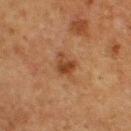<record>
<lesion_size>
  <long_diameter_mm_approx>2.5</long_diameter_mm_approx>
</lesion_size>
<automated_metrics>
  <area_mm2_approx>4.5</area_mm2_approx>
  <eccentricity>0.55</eccentricity>
  <nevus_likeness_0_100>70</nevus_likeness_0_100>
  <lesion_detection_confidence_0_100>100</lesion_detection_confidence_0_100>
</automated_metrics>
<patient>
  <sex>male</sex>
  <age_approx>75</age_approx>
</patient>
<lighting>cross-polarized</lighting>
<image>
  <source>total-body photography crop</source>
  <field_of_view_mm>15</field_of_view_mm>
</image>
<site>upper back</site>
</record>